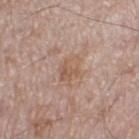Recorded during total-body skin imaging; not selected for excision or biopsy. Approximately 3 mm at its widest. Automated tile analysis of the lesion measured a footprint of about 5.5 mm², a shape eccentricity near 0.65, and a shape-asymmetry score of about 0.25 (0 = symmetric). It also reported an average lesion color of about L≈56 a*≈18 b*≈28 (CIELAB), about 7 CIELAB-L* units darker than the surrounding skin, and a normalized border contrast of about 5.5. It also reported a classifier nevus-likeness of about 0/100. This is a white-light tile. Cropped from a whole-body photographic skin survey; the tile spans about 15 mm. Located on the left thigh. A male patient, aged 53–57.